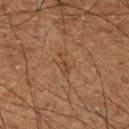workup: imaged on a skin check; not biopsied
acquisition: total-body-photography crop, ~15 mm field of view
lighting: cross-polarized
automated lesion analysis: an area of roughly 3 mm², an eccentricity of roughly 0.85, and a shape-asymmetry score of about 0.45 (0 = symmetric); roughly 5 lightness units darker than nearby skin and a normalized lesion–skin contrast near 5; border irregularity of about 5 on a 0–10 scale, a within-lesion color-variation index near 1.5/10, and peripheral color asymmetry of about 0.5
patient: male, aged approximately 60
location: the right lower leg
lesion size: about 2.5 mm